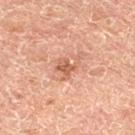Imaged during a routine full-body skin examination; the lesion was not biopsied and no histopathology is available.
A region of skin cropped from a whole-body photographic capture, roughly 15 mm wide.
The patient is a male approximately 65 years of age.
Longest diameter approximately 3.5 mm.
The lesion is located on the left lower leg.
The tile uses cross-polarized illumination.